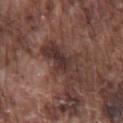Q: Is there a histopathology result?
A: total-body-photography surveillance lesion; no biopsy
Q: What did automated image analysis measure?
A: a border-irregularity index near 6.5/10, internal color variation of about 4 on a 0–10 scale, and radial color variation of about 1.5; a nevus-likeness score of about 0/100 and a detector confidence of about 70 out of 100 that the crop contains a lesion
Q: How was the tile lit?
A: white-light illumination
Q: Where on the body is the lesion?
A: the chest
Q: Lesion size?
A: ≈6 mm
Q: How was this image acquired?
A: ~15 mm tile from a whole-body skin photo
Q: Patient demographics?
A: male, aged approximately 75